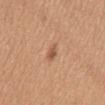No biopsy was performed on this lesion — it was imaged during a full skin examination and was not determined to be concerning.
Imaged with white-light lighting.
About 2.5 mm across.
A 15 mm crop from a total-body photograph taken for skin-cancer surveillance.
Located on the mid back.
A female subject aged 38 to 42.